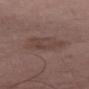Findings:
* workup · total-body-photography surveillance lesion; no biopsy